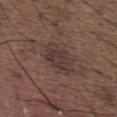biopsy status = catalogued during a skin exam; not biopsied
image source = ~15 mm tile from a whole-body skin photo
lesion diameter = ≈4 mm
patient = male, aged approximately 50
anatomic site = the back